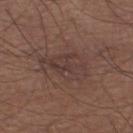{"patient": {"sex": "male", "age_approx": 65}, "lesion_size": {"long_diameter_mm_approx": 5.5}, "site": "left lower leg", "image": {"source": "total-body photography crop", "field_of_view_mm": 15}}